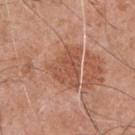Recorded during total-body skin imaging; not selected for excision or biopsy. The lesion-visualizer software estimated a footprint of about 13 mm², an outline eccentricity of about 0.65 (0 = round, 1 = elongated), and a shape-asymmetry score of about 0.4 (0 = symmetric). The analysis additionally found an average lesion color of about L≈53 a*≈24 b*≈32 (CIELAB), a lesion–skin lightness drop of about 9, and a lesion-to-skin contrast of about 6.5 (normalized; higher = more distinct). And it measured a border-irregularity index near 4.5/10 and a color-variation rating of about 3/10. It also reported a detector confidence of about 100 out of 100 that the crop contains a lesion. Captured under white-light illumination. On the chest. A region of skin cropped from a whole-body photographic capture, roughly 15 mm wide. A male subject, in their mid- to late 50s. Longest diameter approximately 5 mm.